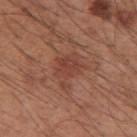Assessment: The lesion was tiled from a total-body skin photograph and was not biopsied. Clinical summary: Longest diameter approximately 4 mm. Located on the right upper arm. A male patient roughly 60 years of age. A 15 mm crop from a total-body photograph taken for skin-cancer surveillance. Captured under white-light illumination.